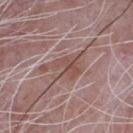<case>
<biopsy_status>not biopsied; imaged during a skin examination</biopsy_status>
</case>